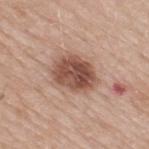Clinical impression:
The lesion was tiled from a total-body skin photograph and was not biopsied.
Context:
A region of skin cropped from a whole-body photographic capture, roughly 15 mm wide. Measured at roughly 5 mm in maximum diameter. A male subject in their mid- to late 60s. Automated tile analysis of the lesion measured an area of roughly 15 mm², an outline eccentricity of about 0.65 (0 = round, 1 = elongated), and a symmetry-axis asymmetry near 0.15. And it measured a mean CIELAB color near L≈51 a*≈21 b*≈27, a lesion–skin lightness drop of about 15, and a normalized lesion–skin contrast near 10. The analysis additionally found an automated nevus-likeness rating near 90 out of 100 and a detector confidence of about 100 out of 100 that the crop contains a lesion. From the upper back.